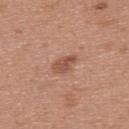notes — catalogued during a skin exam; not biopsied | size — ~3 mm (longest diameter) | image — ~15 mm crop, total-body skin-cancer survey | automated lesion analysis — an area of roughly 4.5 mm² and a shape eccentricity near 0.8; a border-irregularity rating of about 2.5/10, a within-lesion color-variation index near 2/10, and peripheral color asymmetry of about 0.5; a classifier nevus-likeness of about 40/100 | illumination — white-light illumination | subject — female, aged 38–42 | site — the upper back.